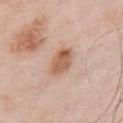{
  "biopsy_status": "not biopsied; imaged during a skin examination",
  "patient": {
    "sex": "male",
    "age_approx": 55
  },
  "automated_metrics": {
    "area_mm2_approx": 7.5,
    "eccentricity": 0.7,
    "shape_asymmetry": 0.15,
    "nevus_likeness_0_100": 70
  },
  "lesion_size": {
    "long_diameter_mm_approx": 3.5
  },
  "site": "chest",
  "lighting": "white-light",
  "image": {
    "source": "total-body photography crop",
    "field_of_view_mm": 15
  }
}